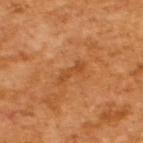Impression:
Recorded during total-body skin imaging; not selected for excision or biopsy.